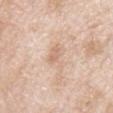{
  "biopsy_status": "not biopsied; imaged during a skin examination",
  "lesion_size": {
    "long_diameter_mm_approx": 4.0
  },
  "patient": {
    "sex": "male",
    "age_approx": 65
  },
  "image": {
    "source": "total-body photography crop",
    "field_of_view_mm": 15
  },
  "site": "chest"
}